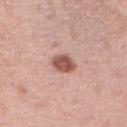This lesion was catalogued during total-body skin photography and was not selected for biopsy. A 15 mm close-up extracted from a 3D total-body photography capture. The total-body-photography lesion software estimated an area of roughly 5.5 mm², an outline eccentricity of about 0.6 (0 = round, 1 = elongated), and two-axis asymmetry of about 0.15. The software also gave a lesion color around L≈54 a*≈24 b*≈26 in CIELAB, about 15 CIELAB-L* units darker than the surrounding skin, and a normalized lesion–skin contrast near 10. The lesion's longest dimension is about 3 mm. The lesion is located on the right lower leg. A female patient, roughly 40 years of age. This is a white-light tile.